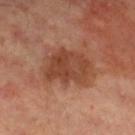This lesion was catalogued during total-body skin photography and was not selected for biopsy.
A 15 mm crop from a total-body photograph taken for skin-cancer surveillance.
A male patient, approximately 50 years of age.
From the left leg.
Imaged with cross-polarized lighting.
About 5.5 mm across.